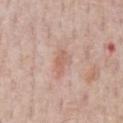Q: What did automated image analysis measure?
A: a lesion color around L≈61 a*≈22 b*≈27 in CIELAB; a nevus-likeness score of about 5/100 and lesion-presence confidence of about 100/100
Q: Lesion location?
A: the chest
Q: How large is the lesion?
A: about 3 mm
Q: Who is the patient?
A: male, aged around 60
Q: What kind of image is this?
A: ~15 mm tile from a whole-body skin photo